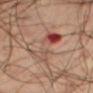Captured during whole-body skin photography for melanoma surveillance; the lesion was not biopsied.
The lesion-visualizer software estimated a mean CIELAB color near L≈48 a*≈22 b*≈26. The analysis additionally found a within-lesion color-variation index near 10/10.
Captured under cross-polarized illumination.
The recorded lesion diameter is about 6.5 mm.
On the right thigh.
A male subject, aged around 60.
Cropped from a whole-body photographic skin survey; the tile spans about 15 mm.